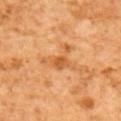Q: Was this lesion biopsied?
A: total-body-photography surveillance lesion; no biopsy
Q: Who is the patient?
A: male, aged around 60
Q: How large is the lesion?
A: about 4.5 mm
Q: How was this image acquired?
A: total-body-photography crop, ~15 mm field of view
Q: Lesion location?
A: the upper back
Q: Illumination type?
A: cross-polarized illumination